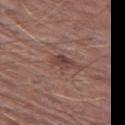The lesion was photographed on a routine skin check and not biopsied; there is no pathology result.
Captured under white-light illumination.
The total-body-photography lesion software estimated a mean CIELAB color near L≈42 a*≈20 b*≈22 and a lesion-to-skin contrast of about 7.5 (normalized; higher = more distinct). The software also gave a color-variation rating of about 3/10 and radial color variation of about 1.
Located on the left thigh.
A male patient, about 75 years old.
A lesion tile, about 15 mm wide, cut from a 3D total-body photograph.